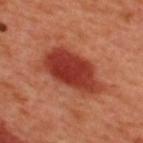Captured during whole-body skin photography for melanoma surveillance; the lesion was not biopsied. This is a cross-polarized tile. A male subject, approximately 50 years of age. An algorithmic analysis of the crop reported an automated nevus-likeness rating near 100 out of 100 and a lesion-detection confidence of about 100/100. A 15 mm close-up extracted from a 3D total-body photography capture. On the upper back.